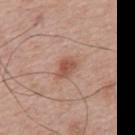<tbp_lesion>
  <biopsy_status>not biopsied; imaged during a skin examination</biopsy_status>
  <automated_metrics>
    <cielab_L>54</cielab_L>
    <cielab_a>23</cielab_a>
    <cielab_b>28</cielab_b>
    <vs_skin_darker_L>10.0</vs_skin_darker_L>
    <vs_skin_contrast_norm>7.0</vs_skin_contrast_norm>
  </automated_metrics>
  <lesion_size>
    <long_diameter_mm_approx>3.5</long_diameter_mm_approx>
  </lesion_size>
  <lighting>white-light</lighting>
  <patient>
    <sex>male</sex>
    <age_approx>65</age_approx>
  </patient>
  <image>
    <source>total-body photography crop</source>
    <field_of_view_mm>15</field_of_view_mm>
  </image>
  <site>mid back</site>
</tbp_lesion>